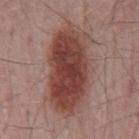{
  "biopsy_status": "not biopsied; imaged during a skin examination",
  "patient": {
    "sex": "male",
    "age_approx": 65
  },
  "image": {
    "source": "total-body photography crop",
    "field_of_view_mm": 15
  },
  "site": "mid back"
}